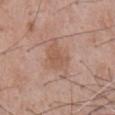Q: Was this lesion biopsied?
A: total-body-photography surveillance lesion; no biopsy
Q: How was this image acquired?
A: total-body-photography crop, ~15 mm field of view
Q: How large is the lesion?
A: ≈4.5 mm
Q: Who is the patient?
A: male, aged 48 to 52
Q: What is the anatomic site?
A: the chest